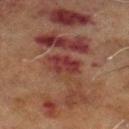biopsy status: no biopsy performed (imaged during a skin exam)
image-analysis metrics: an area of roughly 7.5 mm², a shape eccentricity near 0.7, and a shape-asymmetry score of about 0.2 (0 = symmetric); a lesion-to-skin contrast of about 8.5 (normalized; higher = more distinct); a border-irregularity rating of about 2.5/10, internal color variation of about 3.5 on a 0–10 scale, and peripheral color asymmetry of about 1; a nevus-likeness score of about 0/100 and a detector confidence of about 95 out of 100 that the crop contains a lesion
diameter: about 3.5 mm
body site: the left lower leg
lighting: cross-polarized
image: total-body-photography crop, ~15 mm field of view
patient: male, about 70 years old